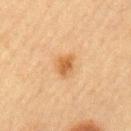Imaged with cross-polarized lighting. Approximately 3 mm at its widest. The lesion-visualizer software estimated border irregularity of about 2.5 on a 0–10 scale, internal color variation of about 2.5 on a 0–10 scale, and a peripheral color-asymmetry measure near 0.5. The software also gave a nevus-likeness score of about 90/100 and lesion-presence confidence of about 100/100. A 15 mm close-up extracted from a 3D total-body photography capture. The lesion is located on the right upper arm. A male patient, in their mid- to late 60s.